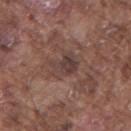– follow-up · no biopsy performed (imaged during a skin exam)
– subject · male, about 75 years old
– image-analysis metrics · an eccentricity of roughly 0.55 and a symmetry-axis asymmetry near 0.3; an average lesion color of about L≈39 a*≈17 b*≈20 (CIELAB), a lesion–skin lightness drop of about 8, and a normalized lesion–skin contrast near 7; internal color variation of about 4.5 on a 0–10 scale and radial color variation of about 1.5; a nevus-likeness score of about 0/100 and lesion-presence confidence of about 95/100
– site · the right upper arm
– image · ~15 mm crop, total-body skin-cancer survey